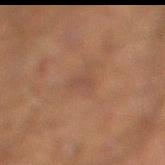Q: Is there a histopathology result?
A: catalogued during a skin exam; not biopsied
Q: Lesion size?
A: ≈2.5 mm
Q: How was the tile lit?
A: cross-polarized illumination
Q: What are the patient's age and sex?
A: male, aged approximately 45
Q: Automated lesion metrics?
A: a lesion color around L≈38 a*≈17 b*≈24 in CIELAB, a lesion–skin lightness drop of about 4, and a lesion-to-skin contrast of about 4.5 (normalized; higher = more distinct); a border-irregularity rating of about 4.5/10, internal color variation of about 0 on a 0–10 scale, and peripheral color asymmetry of about 0; an automated nevus-likeness rating near 0 out of 100
Q: How was this image acquired?
A: ~15 mm tile from a whole-body skin photo
Q: What is the anatomic site?
A: the right lower leg